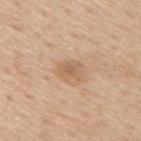<case>
<biopsy_status>not biopsied; imaged during a skin examination</biopsy_status>
<automated_metrics>
  <area_mm2_approx>5.0</area_mm2_approx>
  <eccentricity>0.65</eccentricity>
  <shape_asymmetry>0.3</shape_asymmetry>
  <cielab_L>61</cielab_L>
  <cielab_a>18</cielab_a>
  <cielab_b>33</cielab_b>
  <vs_skin_darker_L>8.0</vs_skin_darker_L>
  <vs_skin_contrast_norm>5.5</vs_skin_contrast_norm>
  <border_irregularity_0_10>3.0</border_irregularity_0_10>
  <color_variation_0_10>3.0</color_variation_0_10>
  <peripheral_color_asymmetry>1.0</peripheral_color_asymmetry>
</automated_metrics>
<image>
  <source>total-body photography crop</source>
  <field_of_view_mm>15</field_of_view_mm>
</image>
<patient>
  <sex>male</sex>
  <age_approx>60</age_approx>
</patient>
<lighting>white-light</lighting>
<lesion_size>
  <long_diameter_mm_approx>2.5</long_diameter_mm_approx>
</lesion_size>
<site>upper back</site>
</case>